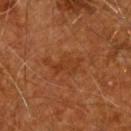{
  "biopsy_status": "not biopsied; imaged during a skin examination",
  "automated_metrics": {
    "border_irregularity_0_10": 5.5,
    "color_variation_0_10": 2.5
  },
  "lighting": "cross-polarized",
  "lesion_size": {
    "long_diameter_mm_approx": 4.5
  },
  "site": "back",
  "patient": {
    "sex": "male",
    "age_approx": 60
  },
  "image": {
    "source": "total-body photography crop",
    "field_of_view_mm": 15
  }
}